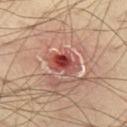Captured during whole-body skin photography for melanoma surveillance; the lesion was not biopsied.